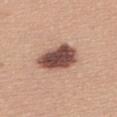Impression:
Recorded during total-body skin imaging; not selected for excision or biopsy.
Image and clinical context:
About 5.5 mm across. This is a white-light tile. The lesion-visualizer software estimated a footprint of about 13 mm². And it measured a border-irregularity rating of about 2/10, a color-variation rating of about 4/10, and radial color variation of about 1. The software also gave a lesion-detection confidence of about 100/100. A close-up tile cropped from a whole-body skin photograph, about 15 mm across. The lesion is on the upper back. The subject is a female approximately 35 years of age.